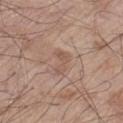size: ≈3.5 mm
imaging modality: ~15 mm tile from a whole-body skin photo
site: the left thigh
illumination: white-light illumination
patient: male, about 80 years old